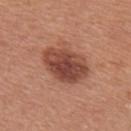Clinical impression: The lesion was tiled from a total-body skin photograph and was not biopsied. Image and clinical context: A 15 mm close-up tile from a total-body photography series done for melanoma screening. A female patient, roughly 45 years of age. Imaged with white-light lighting. An algorithmic analysis of the crop reported a mean CIELAB color near L≈45 a*≈25 b*≈28, about 14 CIELAB-L* units darker than the surrounding skin, and a normalized lesion–skin contrast near 10. And it measured an automated nevus-likeness rating near 70 out of 100 and a detector confidence of about 100 out of 100 that the crop contains a lesion. Located on the upper back. About 5.5 mm across.